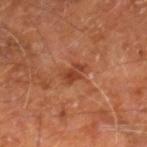notes = no biopsy performed (imaged during a skin exam) | location = the right leg | subject = male, aged around 60 | lighting = cross-polarized | image source = ~15 mm tile from a whole-body skin photo | size = ≈3 mm.